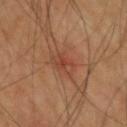This lesion was catalogued during total-body skin photography and was not selected for biopsy.
From the back.
A region of skin cropped from a whole-body photographic capture, roughly 15 mm wide.
A male subject approximately 45 years of age.